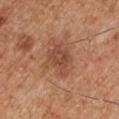– biopsy status: imaged on a skin check; not biopsied
– lesion size: ≈4 mm
– lighting: white-light
– imaging modality: ~15 mm crop, total-body skin-cancer survey
– patient: male, aged 53 to 57
– site: the arm